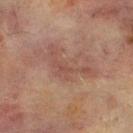Q: Was a biopsy performed?
A: catalogued during a skin exam; not biopsied
Q: What kind of image is this?
A: 15 mm crop, total-body photography
Q: Who is the patient?
A: male, in their 70s
Q: Illumination type?
A: cross-polarized
Q: What is the anatomic site?
A: the front of the torso